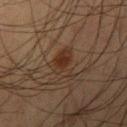Assessment: No biopsy was performed on this lesion — it was imaged during a full skin examination and was not determined to be concerning. Background: This is a cross-polarized tile. The lesion is on the arm. About 3.5 mm across. A region of skin cropped from a whole-body photographic capture, roughly 15 mm wide. A male subject, roughly 55 years of age.